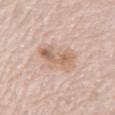{"biopsy_status": "not biopsied; imaged during a skin examination", "lesion_size": {"long_diameter_mm_approx": 4.5}, "image": {"source": "total-body photography crop", "field_of_view_mm": 15}, "lighting": "white-light", "site": "front of the torso", "patient": {"sex": "male", "age_approx": 80}}